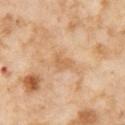follow-up: catalogued during a skin exam; not biopsied.